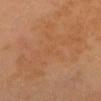Clinical impression: No biopsy was performed on this lesion — it was imaged during a full skin examination and was not determined to be concerning. Background: A 15 mm close-up tile from a total-body photography series done for melanoma screening. A female patient, aged 23–27. Captured under cross-polarized illumination. The recorded lesion diameter is about 14 mm. The lesion is located on the head or neck. The total-body-photography lesion software estimated an automated nevus-likeness rating near 0 out of 100 and a detector confidence of about 100 out of 100 that the crop contains a lesion.